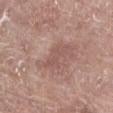Case summary:
– follow-up — imaged on a skin check; not biopsied
– lesion size — about 4 mm
– anatomic site — the abdomen
– patient — male, aged around 80
– acquisition — ~15 mm crop, total-body skin-cancer survey
– lighting — white-light